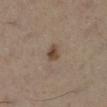Part of a total-body skin-imaging series; this lesion was reviewed on a skin check and was not flagged for biopsy. A female patient, about 50 years old. A roughly 15 mm field-of-view crop from a total-body skin photograph. On the right lower leg.